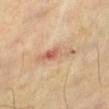biopsy status: catalogued during a skin exam; not biopsied